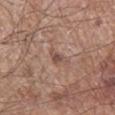image-analysis metrics: a lesion area of about 3.5 mm², an eccentricity of roughly 0.85, and a symmetry-axis asymmetry near 0.45; a border-irregularity index near 5/10, internal color variation of about 1.5 on a 0–10 scale, and radial color variation of about 0.5
site: the right lower leg
image: total-body-photography crop, ~15 mm field of view
illumination: white-light
diameter: ~3 mm (longest diameter)
subject: male, roughly 60 years of age